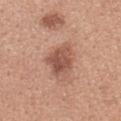Notes:
* workup — no biopsy performed (imaged during a skin exam)
* imaging modality — total-body-photography crop, ~15 mm field of view
* location — the upper back
* subject — female, aged 28 to 32